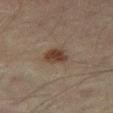Imaged during a routine full-body skin examination; the lesion was not biopsied and no histopathology is available.
The lesion is on the right thigh.
Captured under cross-polarized illumination.
Approximately 3 mm at its widest.
A male subject, aged around 45.
A 15 mm crop from a total-body photograph taken for skin-cancer surveillance.
The lesion-visualizer software estimated an average lesion color of about L≈33 a*≈14 b*≈23 (CIELAB), a lesion–skin lightness drop of about 9, and a lesion-to-skin contrast of about 9 (normalized; higher = more distinct). And it measured a border-irregularity index near 2.5/10 and a color-variation rating of about 2.5/10. The software also gave an automated nevus-likeness rating near 95 out of 100 and a lesion-detection confidence of about 100/100.